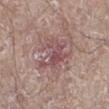Notes:
* notes · no biopsy performed (imaged during a skin exam)
* acquisition · 15 mm crop, total-body photography
* body site · the right lower leg
* diameter · ~4 mm (longest diameter)
* subject · male, aged around 80